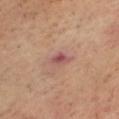This lesion was catalogued during total-body skin photography and was not selected for biopsy. The patient is a male approximately 65 years of age. About 2.5 mm across. From the head or neck. Cropped from a total-body skin-imaging series; the visible field is about 15 mm.